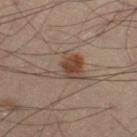{"biopsy_status": "not biopsied; imaged during a skin examination", "patient": {"sex": "male", "age_approx": 50}, "site": "right thigh", "image": {"source": "total-body photography crop", "field_of_view_mm": 15}, "lesion_size": {"long_diameter_mm_approx": 4.5}}